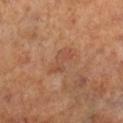Case summary:
• notes · total-body-photography surveillance lesion; no biopsy
• image source · 15 mm crop, total-body photography
• automated metrics · an area of roughly 6 mm², an outline eccentricity of about 0.85 (0 = round, 1 = elongated), and two-axis asymmetry of about 0.4; a nevus-likeness score of about 0/100 and a detector confidence of about 100 out of 100 that the crop contains a lesion
• subject · female, aged 63 to 67
• size · ~3.5 mm (longest diameter)
• anatomic site · the leg
• lighting · cross-polarized illumination